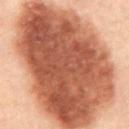workup = catalogued during a skin exam; not biopsied.